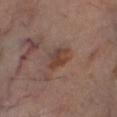Assessment: The lesion was tiled from a total-body skin photograph and was not biopsied. Image and clinical context: Approximately 3.5 mm at its widest. A male subject aged approximately 70. Cropped from a whole-body photographic skin survey; the tile spans about 15 mm. Located on the leg. The tile uses cross-polarized illumination. Automated image analysis of the tile measured a footprint of about 5.5 mm². The analysis additionally found roughly 8 lightness units darker than nearby skin. And it measured a border-irregularity index near 2.5/10. The analysis additionally found an automated nevus-likeness rating near 20 out of 100 and lesion-presence confidence of about 100/100.